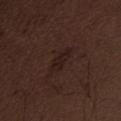{"biopsy_status": "not biopsied; imaged during a skin examination", "image": {"source": "total-body photography crop", "field_of_view_mm": 15}, "lesion_size": {"long_diameter_mm_approx": 4.0}, "site": "abdomen", "patient": {"sex": "male", "age_approx": 70}}